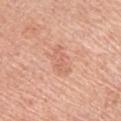Impression:
The lesion was photographed on a routine skin check and not biopsied; there is no pathology result.
Background:
Longest diameter approximately 4 mm. Located on the left upper arm. This image is a 15 mm lesion crop taken from a total-body photograph. A female patient roughly 65 years of age. Imaged with white-light lighting.